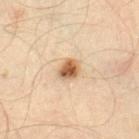Case summary:
– notes: no biopsy performed (imaged during a skin exam)
– patient: male, aged 43 to 47
– illumination: cross-polarized illumination
– anatomic site: the right thigh
– diameter: ~2.5 mm (longest diameter)
– image source: total-body-photography crop, ~15 mm field of view